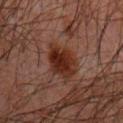Q: How was this image acquired?
A: ~15 mm tile from a whole-body skin photo
Q: What is the lesion's diameter?
A: ≈4.5 mm
Q: What did automated image analysis measure?
A: an area of roughly 11 mm² and a shape eccentricity near 0.7; a classifier nevus-likeness of about 95/100 and a lesion-detection confidence of about 100/100
Q: Where on the body is the lesion?
A: the chest
Q: Illumination type?
A: cross-polarized illumination
Q: Patient demographics?
A: male, in their 60s